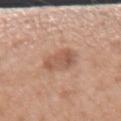This lesion was catalogued during total-body skin photography and was not selected for biopsy. A 15 mm close-up tile from a total-body photography series done for melanoma screening. The subject is a female about 30 years old. On the right forearm.